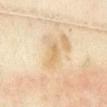Clinical impression: Captured during whole-body skin photography for melanoma surveillance; the lesion was not biopsied. Context: The lesion-visualizer software estimated a border-irregularity index near 5/10 and a peripheral color-asymmetry measure near 0. About 3.5 mm across. The tile uses cross-polarized illumination. A female patient aged 33 to 37. A region of skin cropped from a whole-body photographic capture, roughly 15 mm wide. The lesion is located on the abdomen.